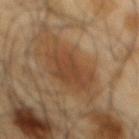Imaged during a routine full-body skin examination; the lesion was not biopsied and no histopathology is available.
Located on the mid back.
Imaged with cross-polarized lighting.
The subject is a male aged around 65.
This image is a 15 mm lesion crop taken from a total-body photograph.
The lesion's longest dimension is about 6.5 mm.
Automated image analysis of the tile measured a border-irregularity index near 3/10, a color-variation rating of about 2.5/10, and radial color variation of about 1. It also reported an automated nevus-likeness rating near 100 out of 100 and a detector confidence of about 95 out of 100 that the crop contains a lesion.